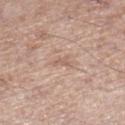A male subject, approximately 55 years of age.
Longest diameter approximately 2.5 mm.
Captured under white-light illumination.
On the right lower leg.
A close-up tile cropped from a whole-body skin photograph, about 15 mm across.